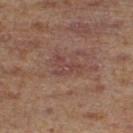Findings:
- notes: total-body-photography surveillance lesion; no biopsy
- image source: 15 mm crop, total-body photography
- location: the left lower leg
- image-analysis metrics: a normalized lesion–skin contrast near 5; a classifier nevus-likeness of about 0/100 and lesion-presence confidence of about 100/100
- diameter: about 3 mm
- patient: male, approximately 65 years of age
- tile lighting: cross-polarized